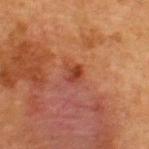Recorded during total-body skin imaging; not selected for excision or biopsy. Cropped from a whole-body photographic skin survey; the tile spans about 15 mm. An algorithmic analysis of the crop reported a mean CIELAB color near L≈36 a*≈27 b*≈31 and a lesion-to-skin contrast of about 7.5 (normalized; higher = more distinct). It also reported border irregularity of about 2.5 on a 0–10 scale, internal color variation of about 3 on a 0–10 scale, and peripheral color asymmetry of about 1. The tile uses cross-polarized illumination. A female subject aged approximately 40. The lesion's longest dimension is about 2 mm. The lesion is located on the upper back.